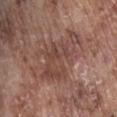Captured during whole-body skin photography for melanoma surveillance; the lesion was not biopsied. Automated image analysis of the tile measured an eccentricity of roughly 0.8 and a shape-asymmetry score of about 0.5 (0 = symmetric). The software also gave an average lesion color of about L≈45 a*≈20 b*≈24 (CIELAB), a lesion–skin lightness drop of about 7, and a lesion-to-skin contrast of about 6 (normalized; higher = more distinct). The recorded lesion diameter is about 7 mm. A male patient, aged around 75. Captured under white-light illumination. Located on the abdomen. Cropped from a whole-body photographic skin survey; the tile spans about 15 mm.